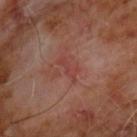Recorded during total-body skin imaging; not selected for excision or biopsy.
A male subject aged around 60.
About 3 mm across.
Located on the chest.
A 15 mm close-up tile from a total-body photography series done for melanoma screening.
The tile uses cross-polarized illumination.